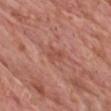This lesion was catalogued during total-body skin photography and was not selected for biopsy.
A male patient in their 60s.
Approximately 2.5 mm at its widest.
A region of skin cropped from a whole-body photographic capture, roughly 15 mm wide.
From the chest.
Captured under white-light illumination.